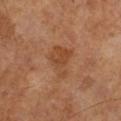Assessment:
Part of a total-body skin-imaging series; this lesion was reviewed on a skin check and was not flagged for biopsy.
Image and clinical context:
About 4 mm across. The lesion is located on the left leg. An algorithmic analysis of the crop reported a nevus-likeness score of about 0/100 and a lesion-detection confidence of about 100/100. A lesion tile, about 15 mm wide, cut from a 3D total-body photograph. This is a cross-polarized tile. A male patient in their mid-60s.